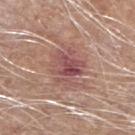Clinical impression: Part of a total-body skin-imaging series; this lesion was reviewed on a skin check and was not flagged for biopsy. Context: The total-body-photography lesion software estimated an outline eccentricity of about 0.5 (0 = round, 1 = elongated). And it measured a border-irregularity index near 5/10, internal color variation of about 7 on a 0–10 scale, and peripheral color asymmetry of about 2.5. It also reported a classifier nevus-likeness of about 5/100 and lesion-presence confidence of about 100/100. A 15 mm close-up extracted from a 3D total-body photography capture. A male patient aged 63–67. Measured at roughly 4.5 mm in maximum diameter. On the right forearm. This is a white-light tile.